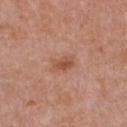The lesion was photographed on a routine skin check and not biopsied; there is no pathology result.
The lesion is located on the chest.
The total-body-photography lesion software estimated an outline eccentricity of about 0.8 (0 = round, 1 = elongated) and two-axis asymmetry of about 0.3. And it measured a lesion color around L≈52 a*≈24 b*≈31 in CIELAB and a lesion-to-skin contrast of about 7 (normalized; higher = more distinct). And it measured a classifier nevus-likeness of about 70/100 and a lesion-detection confidence of about 100/100.
A lesion tile, about 15 mm wide, cut from a 3D total-body photograph.
The subject is a female aged 38 to 42.